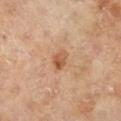A roughly 15 mm field-of-view crop from a total-body skin photograph. This is a cross-polarized tile. The patient is a male aged 63–67. The lesion is located on the right lower leg. Approximately 2.5 mm at its widest. The lesion-visualizer software estimated a nevus-likeness score of about 80/100 and a detector confidence of about 100 out of 100 that the crop contains a lesion.